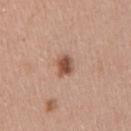biopsy_status: not biopsied; imaged during a skin examination
lesion_size:
  long_diameter_mm_approx: 2.5
image:
  source: total-body photography crop
  field_of_view_mm: 15
site: right upper arm
patient:
  sex: female
  age_approx: 45